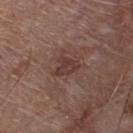biopsy status = imaged on a skin check; not biopsied | lighting = white-light | lesion diameter = ≈3 mm | subject = male, about 80 years old | anatomic site = the front of the torso | imaging modality = ~15 mm crop, total-body skin-cancer survey.